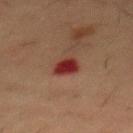Findings:
• patient · male, in their mid-50s
• location · the chest
• image · ~15 mm crop, total-body skin-cancer survey